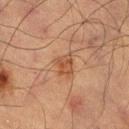Clinical impression:
Part of a total-body skin-imaging series; this lesion was reviewed on a skin check and was not flagged for biopsy.
Image and clinical context:
A 15 mm close-up tile from a total-body photography series done for melanoma screening. A male patient in their mid- to late 60s. From the left thigh.